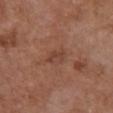Assessment: This lesion was catalogued during total-body skin photography and was not selected for biopsy. Clinical summary: A roughly 15 mm field-of-view crop from a total-body skin photograph. A female patient, roughly 80 years of age. This is a white-light tile. The lesion is located on the chest. Approximately 2.5 mm at its widest.